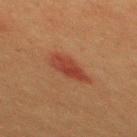Part of a total-body skin-imaging series; this lesion was reviewed on a skin check and was not flagged for biopsy. Longest diameter approximately 4.5 mm. The tile uses cross-polarized illumination. A close-up tile cropped from a whole-body skin photograph, about 15 mm across. A male subject in their 40s. The lesion-visualizer software estimated an area of roughly 8.5 mm², an outline eccentricity of about 0.8 (0 = round, 1 = elongated), and a shape-asymmetry score of about 0.25 (0 = symmetric). And it measured a lesion color around L≈35 a*≈24 b*≈28 in CIELAB, a lesion–skin lightness drop of about 8, and a normalized border contrast of about 7. It also reported internal color variation of about 3.5 on a 0–10 scale and radial color variation of about 1. Located on the mid back.